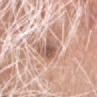<tbp_lesion>
<biopsy_status>not biopsied; imaged during a skin examination</biopsy_status>
<site>head or neck</site>
<image>
  <source>total-body photography crop</source>
  <field_of_view_mm>15</field_of_view_mm>
</image>
<lesion_size>
  <long_diameter_mm_approx>4.5</long_diameter_mm_approx>
</lesion_size>
<lighting>white-light</lighting>
<patient>
  <sex>male</sex>
  <age_approx>80</age_approx>
</patient>
</tbp_lesion>